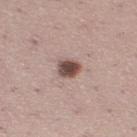Case summary:
* workup · total-body-photography surveillance lesion; no biopsy
* site · the leg
* automated lesion analysis · an outline eccentricity of about 0.45 (0 = round, 1 = elongated) and two-axis asymmetry of about 0.2; a classifier nevus-likeness of about 90/100
* patient · female, aged 23 to 27
* illumination · white-light
* lesion diameter · about 2.5 mm
* acquisition · total-body-photography crop, ~15 mm field of view